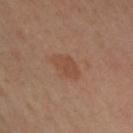{"biopsy_status": "not biopsied; imaged during a skin examination", "image": {"source": "total-body photography crop", "field_of_view_mm": 15}, "patient": {"sex": "female", "age_approx": 50}, "automated_metrics": {"cielab_L": 48, "cielab_a": 21, "cielab_b": 31, "vs_skin_contrast_norm": 5.5, "border_irregularity_0_10": 2.5, "color_variation_0_10": 2.0, "nevus_likeness_0_100": 50}, "site": "arm", "lesion_size": {"long_diameter_mm_approx": 4.0}}